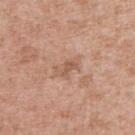Recorded during total-body skin imaging; not selected for excision or biopsy.
A female patient aged approximately 30.
Located on the right upper arm.
The lesion-visualizer software estimated a lesion color around L≈57 a*≈20 b*≈30 in CIELAB and about 8 CIELAB-L* units darker than the surrounding skin. The analysis additionally found a classifier nevus-likeness of about 0/100.
This is a white-light tile.
A roughly 15 mm field-of-view crop from a total-body skin photograph.